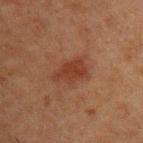The lesion was photographed on a routine skin check and not biopsied; there is no pathology result. Located on the left upper arm. A lesion tile, about 15 mm wide, cut from a 3D total-body photograph. The tile uses cross-polarized illumination. A male subject, aged approximately 50. Measured at roughly 4 mm in maximum diameter.